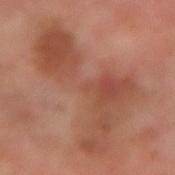Q: Is there a histopathology result?
A: imaged on a skin check; not biopsied
Q: What did automated image analysis measure?
A: a border-irregularity index near 9.5/10, a within-lesion color-variation index near 5.5/10, and radial color variation of about 2; an automated nevus-likeness rating near 0 out of 100 and a lesion-detection confidence of about 100/100
Q: Lesion location?
A: the arm
Q: What kind of image is this?
A: total-body-photography crop, ~15 mm field of view
Q: What are the patient's age and sex?
A: male, about 50 years old
Q: Lesion size?
A: about 12 mm